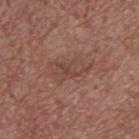Assessment:
The lesion was tiled from a total-body skin photograph and was not biopsied.
Acquisition and patient details:
The lesion is on the upper back. A male patient about 55 years old. The lesion's longest dimension is about 5 mm. Cropped from a whole-body photographic skin survey; the tile spans about 15 mm.